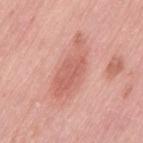workup: catalogued during a skin exam; not biopsied | lesion diameter: ~7.5 mm (longest diameter) | subject: female, aged 38 to 42 | tile lighting: white-light | location: the left thigh | imaging modality: 15 mm crop, total-body photography.